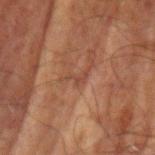Recorded during total-body skin imaging; not selected for excision or biopsy.
Located on the left upper arm.
Approximately 3 mm at its widest.
The lesion-visualizer software estimated an area of roughly 2.5 mm² and an outline eccentricity of about 0.85 (0 = round, 1 = elongated). The analysis additionally found a border-irregularity rating of about 8.5/10, a color-variation rating of about 0/10, and peripheral color asymmetry of about 0.
The patient is a male aged 68–72.
Captured under cross-polarized illumination.
This image is a 15 mm lesion crop taken from a total-body photograph.